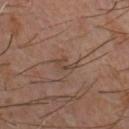Q: How was the tile lit?
A: cross-polarized
Q: Where on the body is the lesion?
A: the chest
Q: Patient demographics?
A: male, in their 60s
Q: How was this image acquired?
A: 15 mm crop, total-body photography
Q: Lesion size?
A: ~2.5 mm (longest diameter)
Q: What did automated image analysis measure?
A: a footprint of about 2.5 mm², an eccentricity of roughly 0.85, and a shape-asymmetry score of about 0.5 (0 = symmetric); a lesion–skin lightness drop of about 6 and a normalized lesion–skin contrast near 5.5; a border-irregularity index near 6.5/10 and a color-variation rating of about 0/10; a nevus-likeness score of about 0/100 and a detector confidence of about 80 out of 100 that the crop contains a lesion